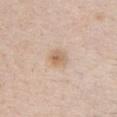Captured during whole-body skin photography for melanoma surveillance; the lesion was not biopsied. From the left upper arm. A lesion tile, about 15 mm wide, cut from a 3D total-body photograph. The lesion's longest dimension is about 2.5 mm. A male patient about 40 years old.